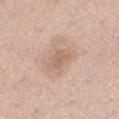Q: Was this lesion biopsied?
A: imaged on a skin check; not biopsied
Q: Lesion size?
A: about 3 mm
Q: How was this image acquired?
A: ~15 mm tile from a whole-body skin photo
Q: Where on the body is the lesion?
A: the right thigh
Q: Who is the patient?
A: male, in their mid-70s
Q: How was the tile lit?
A: white-light illumination